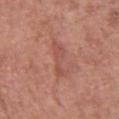Imaged during a routine full-body skin examination; the lesion was not biopsied and no histopathology is available.
A close-up tile cropped from a whole-body skin photograph, about 15 mm across.
The recorded lesion diameter is about 4 mm.
A male subject, aged 53–57.
Captured under white-light illumination.
Located on the chest.